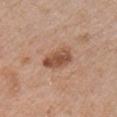Q: Was this lesion biopsied?
A: total-body-photography surveillance lesion; no biopsy
Q: What is the lesion's diameter?
A: ~4 mm (longest diameter)
Q: What are the patient's age and sex?
A: male, aged approximately 65
Q: How was the tile lit?
A: white-light illumination
Q: How was this image acquired?
A: ~15 mm crop, total-body skin-cancer survey
Q: What did automated image analysis measure?
A: a footprint of about 8 mm², an outline eccentricity of about 0.75 (0 = round, 1 = elongated), and a shape-asymmetry score of about 0.2 (0 = symmetric); a detector confidence of about 100 out of 100 that the crop contains a lesion
Q: Lesion location?
A: the front of the torso